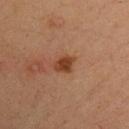Case summary:
* workup: catalogued during a skin exam; not biopsied
* lighting: cross-polarized
* diameter: ≈2.5 mm
* site: the chest
* subject: male, approximately 35 years of age
* acquisition: ~15 mm tile from a whole-body skin photo